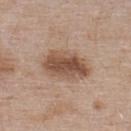Imaged during a routine full-body skin examination; the lesion was not biopsied and no histopathology is available. The recorded lesion diameter is about 5.5 mm. A male patient aged 53 to 57. The tile uses white-light illumination. The total-body-photography lesion software estimated a border-irregularity rating of about 2/10, a within-lesion color-variation index near 5.5/10, and radial color variation of about 2. And it measured a nevus-likeness score of about 85/100 and a detector confidence of about 100 out of 100 that the crop contains a lesion. Located on the upper back. A roughly 15 mm field-of-view crop from a total-body skin photograph.